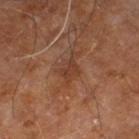- notes · total-body-photography surveillance lesion; no biopsy
- location · the right leg
- imaging modality · ~15 mm crop, total-body skin-cancer survey
- subject · male, aged 58 to 62